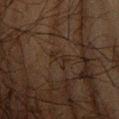Impression:
This lesion was catalogued during total-body skin photography and was not selected for biopsy.
Acquisition and patient details:
A male patient in their 60s. From the right forearm. A lesion tile, about 15 mm wide, cut from a 3D total-body photograph. This is a cross-polarized tile. Longest diameter approximately 2.5 mm.